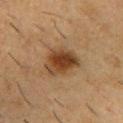The lesion was photographed on a routine skin check and not biopsied; there is no pathology result. Measured at roughly 4.5 mm in maximum diameter. The total-body-photography lesion software estimated a lesion area of about 13 mm², an outline eccentricity of about 0.5 (0 = round, 1 = elongated), and a symmetry-axis asymmetry near 0.25. The software also gave border irregularity of about 2.5 on a 0–10 scale and internal color variation of about 6 on a 0–10 scale. The software also gave an automated nevus-likeness rating near 95 out of 100 and a lesion-detection confidence of about 100/100. The tile uses cross-polarized illumination. A 15 mm close-up tile from a total-body photography series done for melanoma screening. On the upper back. A male subject approximately 55 years of age.